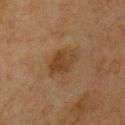This lesion was catalogued during total-body skin photography and was not selected for biopsy. The lesion-visualizer software estimated a classifier nevus-likeness of about 20/100 and lesion-presence confidence of about 100/100. Approximately 4 mm at its widest. From the chest. A close-up tile cropped from a whole-body skin photograph, about 15 mm across. Captured under cross-polarized illumination. The subject is a male aged 73–77.